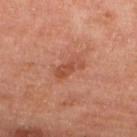biopsy_status: not biopsied; imaged during a skin examination
lighting: cross-polarized
lesion_size:
  long_diameter_mm_approx: 3.5
site: left lower leg
automated_metrics:
  area_mm2_approx: 5.0
  cielab_L: 48
  cielab_a: 26
  cielab_b: 32
  vs_skin_contrast_norm: 6.5
  lesion_detection_confidence_0_100: 100
patient:
  sex: female
  age_approx: 65
image:
  source: total-body photography crop
  field_of_view_mm: 15